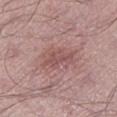Q: What is the imaging modality?
A: ~15 mm tile from a whole-body skin photo
Q: Patient demographics?
A: male, about 55 years old
Q: Lesion location?
A: the left lower leg
Q: Automated lesion metrics?
A: a nevus-likeness score of about 5/100